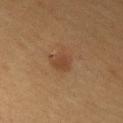<lesion>
  <patient>
    <sex>female</sex>
    <age_approx>50</age_approx>
  </patient>
  <automated_metrics>
    <border_irregularity_0_10>2.5</border_irregularity_0_10>
    <color_variation_0_10>2.5</color_variation_0_10>
    <peripheral_color_asymmetry>1.0</peripheral_color_asymmetry>
  </automated_metrics>
  <lesion_size>
    <long_diameter_mm_approx>3.0</long_diameter_mm_approx>
  </lesion_size>
  <image>
    <source>total-body photography crop</source>
    <field_of_view_mm>15</field_of_view_mm>
  </image>
  <site>front of the torso</site>
</lesion>